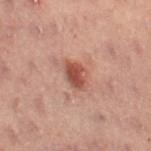Captured during whole-body skin photography for melanoma surveillance; the lesion was not biopsied. A roughly 15 mm field-of-view crop from a total-body skin photograph. A female subject, aged 53–57. Captured under cross-polarized illumination. The total-body-photography lesion software estimated internal color variation of about 2.5 on a 0–10 scale. The lesion is located on the left thigh.